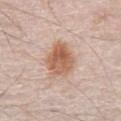Notes:
– workup — catalogued during a skin exam; not biopsied
– subject — male, aged 78–82
– TBP lesion metrics — a footprint of about 13 mm² and a shape eccentricity near 0.55; a mean CIELAB color near L≈60 a*≈21 b*≈31, about 12 CIELAB-L* units darker than the surrounding skin, and a normalized border contrast of about 8.5; a border-irregularity rating of about 2/10 and a within-lesion color-variation index near 5.5/10; an automated nevus-likeness rating near 100 out of 100
– location — the abdomen
– imaging modality — total-body-photography crop, ~15 mm field of view
– lesion diameter — about 4.5 mm
– tile lighting — white-light illumination